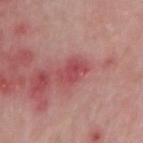Part of a total-body skin-imaging series; this lesion was reviewed on a skin check and was not flagged for biopsy. A male subject, approximately 75 years of age. An algorithmic analysis of the crop reported an area of roughly 6 mm², an outline eccentricity of about 0.8 (0 = round, 1 = elongated), and two-axis asymmetry of about 0.3. The analysis additionally found a lesion color around L≈49 a*≈33 b*≈23 in CIELAB, a lesion–skin lightness drop of about 10, and a normalized border contrast of about 7. The analysis additionally found a border-irregularity index near 3.5/10 and a peripheral color-asymmetry measure near 1. It also reported an automated nevus-likeness rating near 0 out of 100 and a detector confidence of about 100 out of 100 that the crop contains a lesion. This is a white-light tile. The lesion is located on the arm. Measured at roughly 3.5 mm in maximum diameter. A close-up tile cropped from a whole-body skin photograph, about 15 mm across.